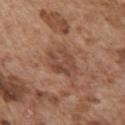| feature | finding |
|---|---|
| notes | total-body-photography surveillance lesion; no biopsy |
| acquisition | 15 mm crop, total-body photography |
| TBP lesion metrics | a footprint of about 7.5 mm² and a symmetry-axis asymmetry near 0.35; a lesion-to-skin contrast of about 6.5 (normalized; higher = more distinct); a classifier nevus-likeness of about 5/100 and a lesion-detection confidence of about 100/100 |
| location | the front of the torso |
| illumination | white-light |
| subject | male, aged around 75 |
| lesion diameter | ~4 mm (longest diameter) |